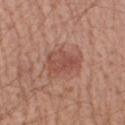workup — imaged on a skin check; not biopsied | automated metrics — a shape eccentricity near 0.7 and a shape-asymmetry score of about 0.3 (0 = symmetric); an average lesion color of about L≈50 a*≈24 b*≈27 (CIELAB), a lesion–skin lightness drop of about 9, and a normalized lesion–skin contrast near 6; a border-irregularity rating of about 3.5/10, internal color variation of about 3.5 on a 0–10 scale, and a peripheral color-asymmetry measure near 1.5; a classifier nevus-likeness of about 90/100 | acquisition — ~15 mm tile from a whole-body skin photo | body site — the right forearm | size — ~4.5 mm (longest diameter) | patient — male, aged around 55.